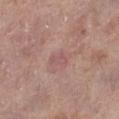No biopsy was performed on this lesion — it was imaged during a full skin examination and was not determined to be concerning. A close-up tile cropped from a whole-body skin photograph, about 15 mm across. The tile uses white-light illumination. The lesion is located on the right lower leg. A female patient approximately 85 years of age or older. Automated image analysis of the tile measured a lesion area of about 2.5 mm² and a symmetry-axis asymmetry near 0.3. The analysis additionally found a normalized lesion–skin contrast near 5. And it measured a border-irregularity rating of about 2.5/10, internal color variation of about 0.5 on a 0–10 scale, and a peripheral color-asymmetry measure near 0. And it measured a classifier nevus-likeness of about 0/100 and a lesion-detection confidence of about 100/100. The recorded lesion diameter is about 2.5 mm.